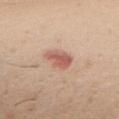Imaged during a routine full-body skin examination; the lesion was not biopsied and no histopathology is available.
A female subject, approximately 50 years of age.
Imaged with white-light lighting.
Measured at roughly 3 mm in maximum diameter.
On the front of the torso.
A lesion tile, about 15 mm wide, cut from a 3D total-body photograph.